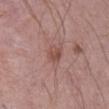Clinical impression:
The lesion was tiled from a total-body skin photograph and was not biopsied.
Acquisition and patient details:
The total-body-photography lesion software estimated an average lesion color of about L≈50 a*≈21 b*≈24 (CIELAB), a lesion–skin lightness drop of about 8, and a lesion-to-skin contrast of about 6 (normalized; higher = more distinct). The analysis additionally found a nevus-likeness score of about 0/100 and a lesion-detection confidence of about 100/100. Cropped from a total-body skin-imaging series; the visible field is about 15 mm. This is a white-light tile. The patient is a male approximately 70 years of age. From the front of the torso.